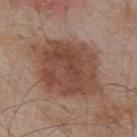Impression:
Recorded during total-body skin imaging; not selected for excision or biopsy.